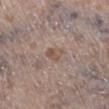A roughly 15 mm field-of-view crop from a total-body skin photograph. A female subject, in their mid-60s. This is a white-light tile. The lesion is located on the right lower leg. Approximately 3 mm at its widest.